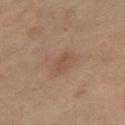notes — no biopsy performed (imaged during a skin exam)
body site — the right thigh
subject — female, approximately 55 years of age
imaging modality — 15 mm crop, total-body photography